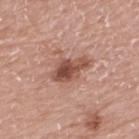Q: How was this image acquired?
A: ~15 mm crop, total-body skin-cancer survey
Q: What are the patient's age and sex?
A: male, about 75 years old
Q: How large is the lesion?
A: about 4.5 mm
Q: Illumination type?
A: white-light illumination
Q: Lesion location?
A: the upper back